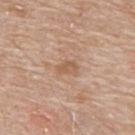  biopsy_status: not biopsied; imaged during a skin examination
  site: back
  lighting: white-light
  image:
    source: total-body photography crop
    field_of_view_mm: 15
  patient:
    sex: male
    age_approx: 65
  lesion_size:
    long_diameter_mm_approx: 2.5